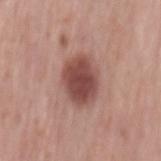site: mid back
image:
  source: total-body photography crop
  field_of_view_mm: 15
patient:
  sex: male
  age_approx: 65